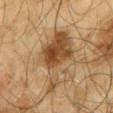The patient is a male in their mid-60s. An algorithmic analysis of the crop reported a lesion area of about 21 mm², an outline eccentricity of about 0.8 (0 = round, 1 = elongated), and a shape-asymmetry score of about 0.5 (0 = symmetric). It also reported a border-irregularity index near 7/10, a color-variation rating of about 5/10, and peripheral color asymmetry of about 1.5. A 15 mm crop from a total-body photograph taken for skin-cancer surveillance. Approximately 8 mm at its widest. On the mid back. Captured under cross-polarized illumination.